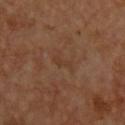The subject is a male in their 60s.
The lesion-visualizer software estimated a border-irregularity rating of about 4.5/10 and a color-variation rating of about 0/10.
Cropped from a whole-body photographic skin survey; the tile spans about 15 mm.
On the upper back.
Imaged with cross-polarized lighting.
Approximately 2.5 mm at its widest.